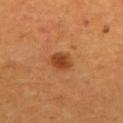Recorded during total-body skin imaging; not selected for excision or biopsy.
Automated image analysis of the tile measured roughly 11 lightness units darker than nearby skin and a lesion-to-skin contrast of about 8.5 (normalized; higher = more distinct). The analysis additionally found a border-irregularity rating of about 2/10, a color-variation rating of about 2.5/10, and peripheral color asymmetry of about 1. The software also gave a classifier nevus-likeness of about 95/100 and a lesion-detection confidence of about 100/100.
A male patient in their 60s.
Located on the mid back.
A region of skin cropped from a whole-body photographic capture, roughly 15 mm wide.